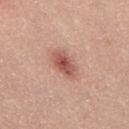Assessment: The lesion was tiled from a total-body skin photograph and was not biopsied. Clinical summary: Imaged with white-light lighting. The patient is a male approximately 25 years of age. A close-up tile cropped from a whole-body skin photograph, about 15 mm across. Approximately 4 mm at its widest. On the mid back.